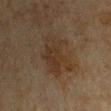workup=no biopsy performed (imaged during a skin exam); acquisition=15 mm crop, total-body photography; subject=male, aged 63 to 67; location=the right upper arm.